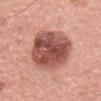Clinical summary: On the head or neck. The tile uses white-light illumination. The recorded lesion diameter is about 6 mm. A female patient, aged approximately 35. A close-up tile cropped from a whole-body skin photograph, about 15 mm across.